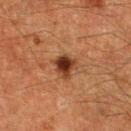| feature | finding |
|---|---|
| workup | no biopsy performed (imaged during a skin exam) |
| diameter | about 3 mm |
| image source | 15 mm crop, total-body photography |
| location | the right lower leg |
| subject | male, in their 60s |
| lighting | cross-polarized |
| image-analysis metrics | an area of roughly 5.5 mm² and a shape-asymmetry score of about 0.25 (0 = symmetric); a mean CIELAB color near L≈28 a*≈20 b*≈27 and a lesion–skin lightness drop of about 13; border irregularity of about 2.5 on a 0–10 scale and a color-variation rating of about 4.5/10 |